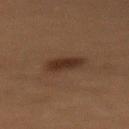Clinical impression:
Imaged during a routine full-body skin examination; the lesion was not biopsied and no histopathology is available.
Context:
The lesion-visualizer software estimated a lesion area of about 6 mm² and two-axis asymmetry of about 0.25. The analysis additionally found a lesion color around L≈22 a*≈14 b*≈21 in CIELAB and a lesion-to-skin contrast of about 9 (normalized; higher = more distinct). The software also gave border irregularity of about 2.5 on a 0–10 scale and a peripheral color-asymmetry measure near 1. The software also gave an automated nevus-likeness rating near 80 out of 100 and a detector confidence of about 100 out of 100 that the crop contains a lesion. This is a cross-polarized tile. On the chest. The patient is a female in their 80s. A roughly 15 mm field-of-view crop from a total-body skin photograph. Measured at roughly 3.5 mm in maximum diameter.